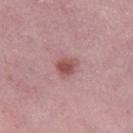Captured during whole-body skin photography for melanoma surveillance; the lesion was not biopsied. The tile uses white-light illumination. From the right lower leg. A region of skin cropped from a whole-body photographic capture, roughly 15 mm wide. A female patient approximately 45 years of age. Approximately 2.5 mm at its widest.